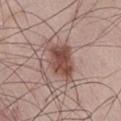notes: total-body-photography surveillance lesion; no biopsy | anatomic site: the right thigh | lighting: white-light illumination | image-analysis metrics: a lesion area of about 13 mm², an eccentricity of roughly 0.8, and two-axis asymmetry of about 0.35; an average lesion color of about L≈49 a*≈20 b*≈24 (CIELAB), a lesion–skin lightness drop of about 12, and a normalized lesion–skin contrast near 9; a border-irregularity index near 3.5/10 and a color-variation rating of about 4/10 | patient: male, roughly 60 years of age | imaging modality: ~15 mm tile from a whole-body skin photo | size: ≈5.5 mm.